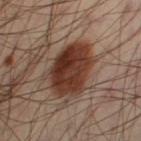Imaged during a routine full-body skin examination; the lesion was not biopsied and no histopathology is available. The patient is a male aged approximately 40. The lesion is located on the left thigh. Cropped from a whole-body photographic skin survey; the tile spans about 15 mm. The tile uses cross-polarized illumination.